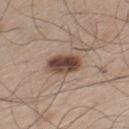biopsy_status: not biopsied; imaged during a skin examination
patient:
  sex: male
  age_approx: 60
lesion_size:
  long_diameter_mm_approx: 4.0
site: leg
lighting: white-light
image:
  source: total-body photography crop
  field_of_view_mm: 15